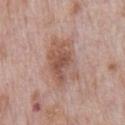Acquisition and patient details: The lesion is on the chest. A 15 mm crop from a total-body photograph taken for skin-cancer surveillance. A male subject, roughly 70 years of age. This is a white-light tile. An algorithmic analysis of the crop reported a lesion area of about 16 mm², an outline eccentricity of about 0.8 (0 = round, 1 = elongated), and a symmetry-axis asymmetry near 0.25. And it measured an automated nevus-likeness rating near 25 out of 100 and a lesion-detection confidence of about 100/100. The lesion's longest dimension is about 6 mm.